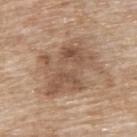Impression: This lesion was catalogued during total-body skin photography and was not selected for biopsy. Acquisition and patient details: A female subject, approximately 75 years of age. Located on the upper back. Cropped from a whole-body photographic skin survey; the tile spans about 15 mm. Approximately 7.5 mm at its widest. Imaged with white-light lighting.